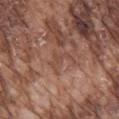Background:
The patient is a male aged approximately 75. Cropped from a whole-body photographic skin survey; the tile spans about 15 mm. From the mid back. This is a white-light tile. Approximately 3.5 mm at its widest.